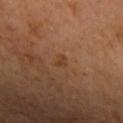- notes · imaged on a skin check; not biopsied
- anatomic site · the arm
- illumination · cross-polarized illumination
- patient · female, aged 38 to 42
- automated lesion analysis · a lesion area of about 1.5 mm², an eccentricity of roughly 0.75, and a symmetry-axis asymmetry near 0.45; border irregularity of about 3.5 on a 0–10 scale, a color-variation rating of about 0/10, and radial color variation of about 0; a nevus-likeness score of about 15/100 and a detector confidence of about 100 out of 100 that the crop contains a lesion
- size · ≈2 mm
- image · ~15 mm tile from a whole-body skin photo